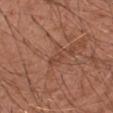Recorded during total-body skin imaging; not selected for excision or biopsy. This image is a 15 mm lesion crop taken from a total-body photograph. The patient is a male aged around 30. Located on the right upper arm. Longest diameter approximately 3 mm. The lesion-visualizer software estimated a lesion area of about 3.5 mm² and a shape eccentricity near 0.8. Captured under white-light illumination.